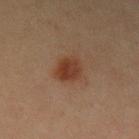This lesion was catalogued during total-body skin photography and was not selected for biopsy.
The lesion is on the left upper arm.
A female patient, aged 38 to 42.
A region of skin cropped from a whole-body photographic capture, roughly 15 mm wide.
This is a cross-polarized tile.